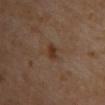Findings:
– image source — 15 mm crop, total-body photography
– lighting — cross-polarized illumination
– image-analysis metrics — a lesion–skin lightness drop of about 8; internal color variation of about 2 on a 0–10 scale and peripheral color asymmetry of about 0.5
– body site — the chest
– diameter — ~2.5 mm (longest diameter)
– patient — female, aged around 50